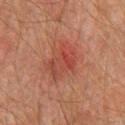Impression:
Part of a total-body skin-imaging series; this lesion was reviewed on a skin check and was not flagged for biopsy.
Clinical summary:
The recorded lesion diameter is about 4.5 mm. A 15 mm close-up tile from a total-body photography series done for melanoma screening. This is a cross-polarized tile. Automated tile analysis of the lesion measured border irregularity of about 4.5 on a 0–10 scale, a color-variation rating of about 6/10, and radial color variation of about 2. A male patient aged 73–77. The lesion is on the chest.